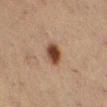{
  "biopsy_status": "not biopsied; imaged during a skin examination",
  "site": "right lower leg",
  "lesion_size": {
    "long_diameter_mm_approx": 3.0
  },
  "image": {
    "source": "total-body photography crop",
    "field_of_view_mm": 15
  },
  "lighting": "cross-polarized",
  "patient": {
    "sex": "female",
    "age_approx": 55
  }
}